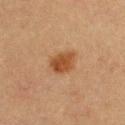biopsy status — no biopsy performed (imaged during a skin exam); anatomic site — the chest; imaging modality — ~15 mm crop, total-body skin-cancer survey; lesion diameter — ≈3.5 mm; illumination — cross-polarized illumination; TBP lesion metrics — a lesion area of about 7 mm² and a shape eccentricity near 0.65; patient — male, aged around 40.